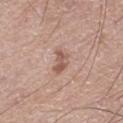Imaged during a routine full-body skin examination; the lesion was not biopsied and no histopathology is available. About 3 mm across. Automated tile analysis of the lesion measured an average lesion color of about L≈55 a*≈20 b*≈26 (CIELAB). The software also gave border irregularity of about 4 on a 0–10 scale, a color-variation rating of about 2/10, and radial color variation of about 0.5. And it measured a classifier nevus-likeness of about 25/100 and a lesion-detection confidence of about 100/100. The patient is a male in their mid-70s. A close-up tile cropped from a whole-body skin photograph, about 15 mm across. The lesion is on the left thigh.